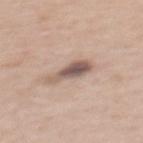Notes:
– workup — catalogued during a skin exam; not biopsied
– patient — female, aged around 40
– diameter — about 4.5 mm
– image source — total-body-photography crop, ~15 mm field of view
– illumination — white-light
– image-analysis metrics — a lesion color around L≈56 a*≈16 b*≈23 in CIELAB, a lesion–skin lightness drop of about 14, and a normalized lesion–skin contrast near 9; a classifier nevus-likeness of about 20/100 and a detector confidence of about 100 out of 100 that the crop contains a lesion
– anatomic site — the mid back